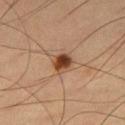Assessment:
Recorded during total-body skin imaging; not selected for excision or biopsy.
Image and clinical context:
A male subject approximately 60 years of age. A close-up tile cropped from a whole-body skin photograph, about 15 mm across. Captured under cross-polarized illumination. From the right thigh.